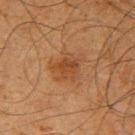Notes:
* workup · imaged on a skin check; not biopsied
* imaging modality · total-body-photography crop, ~15 mm field of view
* size · ~3.5 mm (longest diameter)
* site · the arm
* automated lesion analysis · a mean CIELAB color near L≈37 a*≈20 b*≈31, roughly 7 lightness units darker than nearby skin, and a lesion-to-skin contrast of about 6 (normalized; higher = more distinct); border irregularity of about 3.5 on a 0–10 scale, a within-lesion color-variation index near 3.5/10, and peripheral color asymmetry of about 1
* lighting · cross-polarized illumination
* subject · male, roughly 65 years of age